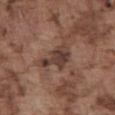Impression:
This lesion was catalogued during total-body skin photography and was not selected for biopsy.
Clinical summary:
A male patient aged 73–77. This image is a 15 mm lesion crop taken from a total-body photograph. The tile uses white-light illumination. Approximately 4.5 mm at its widest. On the abdomen. Automated tile analysis of the lesion measured a lesion area of about 8 mm², an outline eccentricity of about 0.8 (0 = round, 1 = elongated), and two-axis asymmetry of about 0.45. The software also gave a border-irregularity index near 5.5/10, a within-lesion color-variation index near 3.5/10, and a peripheral color-asymmetry measure near 1. The analysis additionally found a nevus-likeness score of about 0/100 and a lesion-detection confidence of about 100/100.